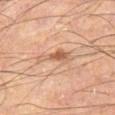  biopsy_status: not biopsied; imaged during a skin examination
  site: left thigh
  image:
    source: total-body photography crop
    field_of_view_mm: 15
  automated_metrics:
    vs_skin_darker_L: 9.0
    vs_skin_contrast_norm: 6.0
    border_irregularity_0_10: 6.0
    color_variation_0_10: 4.5
    peripheral_color_asymmetry: 1.0
  lighting: cross-polarized
  lesion_size:
    long_diameter_mm_approx: 5.0
  patient:
    sex: male
    age_approx: 55